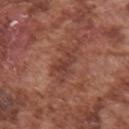Recorded during total-body skin imaging; not selected for excision or biopsy. A roughly 15 mm field-of-view crop from a total-body skin photograph. From the right upper arm. The subject is a male aged around 75.